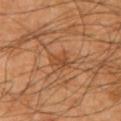follow-up = catalogued during a skin exam; not biopsied
illumination = cross-polarized
patient = male, in their mid- to late 40s
TBP lesion metrics = an automated nevus-likeness rating near 5 out of 100 and a detector confidence of about 100 out of 100 that the crop contains a lesion
location = the left upper arm
acquisition = 15 mm crop, total-body photography